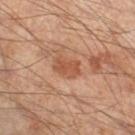workup = imaged on a skin check; not biopsied
image source = 15 mm crop, total-body photography
body site = the right lower leg
patient = male, in their mid- to late 60s
lesion size = ~3 mm (longest diameter)
lighting = cross-polarized